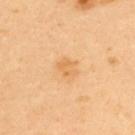Clinical impression:
Captured during whole-body skin photography for melanoma surveillance; the lesion was not biopsied.
Clinical summary:
On the upper back. A male patient, roughly 40 years of age. A close-up tile cropped from a whole-body skin photograph, about 15 mm across. Imaged with cross-polarized lighting. Longest diameter approximately 2.5 mm.